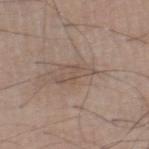{"biopsy_status": "not biopsied; imaged during a skin examination", "patient": {"sex": "male", "age_approx": 20}, "image": {"source": "total-body photography crop", "field_of_view_mm": 15}, "automated_metrics": {"eccentricity": 0.9, "shape_asymmetry": 0.6, "cielab_L": 51, "cielab_a": 14, "cielab_b": 24, "vs_skin_darker_L": 7.0, "nevus_likeness_0_100": 0, "lesion_detection_confidence_0_100": 85}, "lighting": "white-light", "lesion_size": {"long_diameter_mm_approx": 4.0}, "site": "right lower leg"}